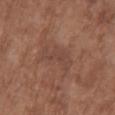Assessment: Part of a total-body skin-imaging series; this lesion was reviewed on a skin check and was not flagged for biopsy.